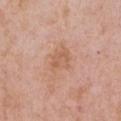Findings:
– workup: imaged on a skin check; not biopsied
– TBP lesion metrics: a lesion color around L≈60 a*≈22 b*≈32 in CIELAB, about 7 CIELAB-L* units darker than the surrounding skin, and a lesion-to-skin contrast of about 5.5 (normalized; higher = more distinct); a color-variation rating of about 2.5/10 and radial color variation of about 0.5
– lighting: white-light illumination
– imaging modality: 15 mm crop, total-body photography
– diameter: about 3 mm
– subject: female, aged 58 to 62
– anatomic site: the chest